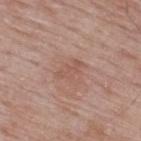The lesion was tiled from a total-body skin photograph and was not biopsied. From the upper back. Captured under white-light illumination. A 15 mm close-up extracted from a 3D total-body photography capture. Longest diameter approximately 3.5 mm. A male subject, aged around 65.